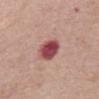Assessment: Captured during whole-body skin photography for melanoma surveillance; the lesion was not biopsied. Image and clinical context: The tile uses white-light illumination. A female subject, aged approximately 75. The lesion's longest dimension is about 3.5 mm. The total-body-photography lesion software estimated roughly 17 lightness units darker than nearby skin and a normalized lesion–skin contrast near 12. The analysis additionally found a classifier nevus-likeness of about 0/100. The lesion is on the front of the torso. A lesion tile, about 15 mm wide, cut from a 3D total-body photograph.